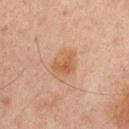The lesion was photographed on a routine skin check and not biopsied; there is no pathology result. The patient is a male aged 63–67. Measured at roughly 4 mm in maximum diameter. This image is a 15 mm lesion crop taken from a total-body photograph. The tile uses cross-polarized illumination. The lesion is located on the mid back. An algorithmic analysis of the crop reported a border-irregularity index near 2/10, a within-lesion color-variation index near 4.5/10, and peripheral color asymmetry of about 1.5. And it measured a detector confidence of about 100 out of 100 that the crop contains a lesion.